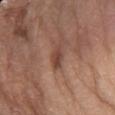biopsy status: total-body-photography surveillance lesion; no biopsy
body site: the arm
image-analysis metrics: a mean CIELAB color near L≈44 a*≈21 b*≈27, roughly 9 lightness units darker than nearby skin, and a normalized border contrast of about 7; a border-irregularity rating of about 4/10 and a within-lesion color-variation index near 1.5/10; lesion-presence confidence of about 90/100
subject: female, roughly 75 years of age
lesion diameter: ≈3 mm
tile lighting: white-light illumination
acquisition: total-body-photography crop, ~15 mm field of view